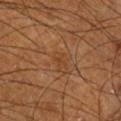Clinical impression:
The lesion was photographed on a routine skin check and not biopsied; there is no pathology result.
Clinical summary:
The subject is a male aged 68 to 72. The lesion-visualizer software estimated a lesion area of about 3 mm², a shape eccentricity near 0.85, and a shape-asymmetry score of about 0.4 (0 = symmetric). The analysis additionally found a classifier nevus-likeness of about 0/100 and a detector confidence of about 95 out of 100 that the crop contains a lesion. The lesion is located on the right thigh. The lesion's longest dimension is about 2.5 mm. Captured under cross-polarized illumination. A roughly 15 mm field-of-view crop from a total-body skin photograph.